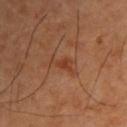Part of a total-body skin-imaging series; this lesion was reviewed on a skin check and was not flagged for biopsy.
Captured under cross-polarized illumination.
A 15 mm close-up extracted from a 3D total-body photography capture.
The recorded lesion diameter is about 3 mm.
The patient is a male roughly 45 years of age.
On the upper back.